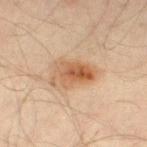Q: Was this lesion biopsied?
A: catalogued during a skin exam; not biopsied
Q: Illumination type?
A: cross-polarized
Q: What is the lesion's diameter?
A: about 5 mm
Q: Where on the body is the lesion?
A: the leg
Q: How was this image acquired?
A: total-body-photography crop, ~15 mm field of view
Q: What are the patient's age and sex?
A: male, aged 43 to 47